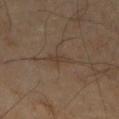Part of a total-body skin-imaging series; this lesion was reviewed on a skin check and was not flagged for biopsy. A roughly 15 mm field-of-view crop from a total-body skin photograph. Imaged with cross-polarized lighting. A male subject roughly 70 years of age. The lesion is located on the left lower leg. The lesion's longest dimension is about 3.5 mm.